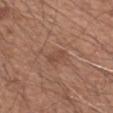Assessment: Imaged during a routine full-body skin examination; the lesion was not biopsied and no histopathology is available. Acquisition and patient details: Imaged with white-light lighting. A male subject, aged 48 to 52. Automated image analysis of the tile measured roughly 7 lightness units darker than nearby skin and a normalized lesion–skin contrast near 5. And it measured an automated nevus-likeness rating near 5 out of 100. A close-up tile cropped from a whole-body skin photograph, about 15 mm across. On the right forearm.